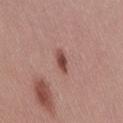Impression: Captured during whole-body skin photography for melanoma surveillance; the lesion was not biopsied. Context: The recorded lesion diameter is about 3 mm. A close-up tile cropped from a whole-body skin photograph, about 15 mm across. The lesion-visualizer software estimated a lesion color around L≈47 a*≈23 b*≈25 in CIELAB, roughly 13 lightness units darker than nearby skin, and a normalized lesion–skin contrast near 9.5. And it measured a border-irregularity index near 2.5/10, internal color variation of about 1.5 on a 0–10 scale, and peripheral color asymmetry of about 0.5. A female patient about 25 years old. The lesion is on the lower back. This is a white-light tile.